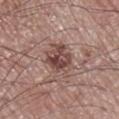This lesion was catalogued during total-body skin photography and was not selected for biopsy.
A lesion tile, about 15 mm wide, cut from a 3D total-body photograph.
The lesion is located on the leg.
Captured under white-light illumination.
A male subject roughly 70 years of age.